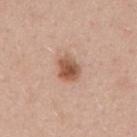The lesion was photographed on a routine skin check and not biopsied; there is no pathology result.
A lesion tile, about 15 mm wide, cut from a 3D total-body photograph.
Automated image analysis of the tile measured an area of roughly 6.5 mm² and a shape-asymmetry score of about 0.2 (0 = symmetric). And it measured a nevus-likeness score of about 95/100.
A female subject aged approximately 40.
Measured at roughly 3 mm in maximum diameter.
Captured under white-light illumination.
From the mid back.